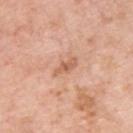notes — catalogued during a skin exam; not biopsied
body site — the upper back
patient — male, roughly 60 years of age
TBP lesion metrics — an average lesion color of about L≈61 a*≈24 b*≈34 (CIELAB), a lesion–skin lightness drop of about 10, and a normalized lesion–skin contrast near 6.5; border irregularity of about 3 on a 0–10 scale and peripheral color asymmetry of about 0
diameter — ≈3 mm
lighting — white-light illumination
acquisition — total-body-photography crop, ~15 mm field of view